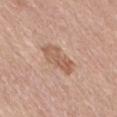Recorded during total-body skin imaging; not selected for excision or biopsy.
About 4.5 mm across.
Located on the right thigh.
A female subject, aged 63–67.
This is a white-light tile.
The lesion-visualizer software estimated an average lesion color of about L≈58 a*≈20 b*≈30 (CIELAB), a lesion–skin lightness drop of about 9, and a lesion-to-skin contrast of about 7 (normalized; higher = more distinct). And it measured a lesion-detection confidence of about 100/100.
A 15 mm crop from a total-body photograph taken for skin-cancer surveillance.